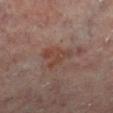Impression:
Captured during whole-body skin photography for melanoma surveillance; the lesion was not biopsied.
Background:
An algorithmic analysis of the crop reported a mean CIELAB color near L≈40 a*≈19 b*≈24, about 6 CIELAB-L* units darker than the surrounding skin, and a normalized border contrast of about 6.5. The tile uses cross-polarized illumination. A 15 mm close-up tile from a total-body photography series done for melanoma screening. A male patient aged 63–67. On the right lower leg.